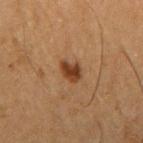| feature | finding |
|---|---|
| workup | catalogued during a skin exam; not biopsied |
| illumination | cross-polarized illumination |
| patient | male, aged approximately 65 |
| automated lesion analysis | a lesion area of about 4.5 mm² and a shape-asymmetry score of about 0.3 (0 = symmetric); an average lesion color of about L≈33 a*≈19 b*≈29 (CIELAB) and a normalized border contrast of about 10; border irregularity of about 2.5 on a 0–10 scale, a color-variation rating of about 3/10, and peripheral color asymmetry of about 1; an automated nevus-likeness rating near 95 out of 100 and lesion-presence confidence of about 100/100 |
| anatomic site | the right upper arm |
| image | 15 mm crop, total-body photography |